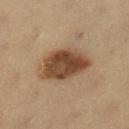Imaged during a routine full-body skin examination; the lesion was not biopsied and no histopathology is available.
The patient is a female in their 40s.
The lesion is located on the leg.
The tile uses cross-polarized illumination.
Longest diameter approximately 6 mm.
This image is a 15 mm lesion crop taken from a total-body photograph.